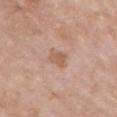Clinical impression:
This lesion was catalogued during total-body skin photography and was not selected for biopsy.
Clinical summary:
About 2.5 mm across. On the chest. A female patient, about 75 years old. Imaged with white-light lighting. The total-body-photography lesion software estimated a footprint of about 4.5 mm² and an eccentricity of roughly 0.6. And it measured an average lesion color of about L≈59 a*≈20 b*≈29 (CIELAB), about 8 CIELAB-L* units darker than the surrounding skin, and a lesion-to-skin contrast of about 6 (normalized; higher = more distinct). The analysis additionally found a border-irregularity index near 2.5/10, a color-variation rating of about 1.5/10, and peripheral color asymmetry of about 0.5. The analysis additionally found an automated nevus-likeness rating near 5 out of 100. Cropped from a whole-body photographic skin survey; the tile spans about 15 mm.